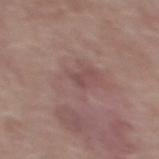This is a white-light tile.
The patient is a female aged 63–67.
A 15 mm close-up tile from a total-body photography series done for melanoma screening.
Measured at roughly 1 mm in maximum diameter.
From the upper back.
Biopsy histopathology demonstrated a melanoma in situ (malignant).